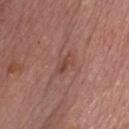notes: catalogued during a skin exam; not biopsied | subject: male, in their 60s | site: the chest | diameter: ≈3 mm | TBP lesion metrics: a mean CIELAB color near L≈45 a*≈23 b*≈26 and roughly 7 lightness units darker than nearby skin; a border-irregularity rating of about 5/10 and internal color variation of about 0.5 on a 0–10 scale | imaging modality: 15 mm crop, total-body photography | illumination: white-light.